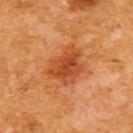Recorded during total-body skin imaging; not selected for excision or biopsy.
A female subject, in their mid-50s.
The lesion-visualizer software estimated roughly 11 lightness units darker than nearby skin and a normalized border contrast of about 7.5.
Located on the upper back.
The lesion's longest dimension is about 4.5 mm.
A 15 mm close-up tile from a total-body photography series done for melanoma screening.
Imaged with cross-polarized lighting.